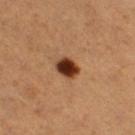{
  "biopsy_status": "not biopsied; imaged during a skin examination",
  "site": "right thigh",
  "patient": {
    "sex": "female",
    "age_approx": 55
  },
  "image": {
    "source": "total-body photography crop",
    "field_of_view_mm": 15
  }
}